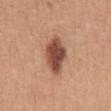Impression: The lesion was photographed on a routine skin check and not biopsied; there is no pathology result. Image and clinical context: A close-up tile cropped from a whole-body skin photograph, about 15 mm across. A female patient aged 38–42. The total-body-photography lesion software estimated a lesion-to-skin contrast of about 11 (normalized; higher = more distinct). And it measured border irregularity of about 2.5 on a 0–10 scale, a color-variation rating of about 4.5/10, and a peripheral color-asymmetry measure near 1.5. The software also gave an automated nevus-likeness rating near 95 out of 100 and a lesion-detection confidence of about 100/100. Approximately 5 mm at its widest. The tile uses white-light illumination. On the abdomen.